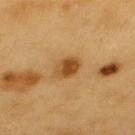Part of a total-body skin-imaging series; this lesion was reviewed on a skin check and was not flagged for biopsy. Cropped from a total-body skin-imaging series; the visible field is about 15 mm. The lesion is located on the upper back. Longest diameter approximately 3.5 mm. The tile uses cross-polarized illumination. A male patient, about 60 years old.